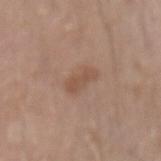{
  "biopsy_status": "not biopsied; imaged during a skin examination",
  "lighting": "white-light",
  "image": {
    "source": "total-body photography crop",
    "field_of_view_mm": 15
  },
  "automated_metrics": {
    "area_mm2_approx": 5.5,
    "nevus_likeness_0_100": 10,
    "lesion_detection_confidence_0_100": 100
  },
  "site": "left forearm",
  "patient": {
    "sex": "male",
    "age_approx": 40
  }
}